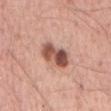subject: male, about 55 years old; lighting: white-light; size: about 4 mm; location: the mid back; image: total-body-photography crop, ~15 mm field of view.